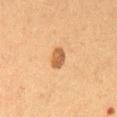Case summary:
– workup: total-body-photography surveillance lesion; no biopsy
– image-analysis metrics: an area of roughly 4.5 mm², a shape eccentricity near 0.7, and two-axis asymmetry of about 0.25; a border-irregularity rating of about 2/10, internal color variation of about 3 on a 0–10 scale, and peripheral color asymmetry of about 1; a nevus-likeness score of about 85/100 and a lesion-detection confidence of about 100/100
– lighting: cross-polarized illumination
– image source: total-body-photography crop, ~15 mm field of view
– subject: female, aged approximately 50
– anatomic site: the abdomen